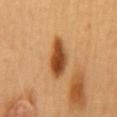No biopsy was performed on this lesion — it was imaged during a full skin examination and was not determined to be concerning.
The total-body-photography lesion software estimated a mean CIELAB color near L≈50 a*≈27 b*≈42 and a lesion-to-skin contrast of about 12 (normalized; higher = more distinct). The software also gave a border-irregularity index near 2/10 and a color-variation rating of about 5.5/10. The software also gave a nevus-likeness score of about 95/100 and a detector confidence of about 100 out of 100 that the crop contains a lesion.
The recorded lesion diameter is about 5 mm.
A female patient approximately 60 years of age.
Imaged with cross-polarized lighting.
Located on the mid back.
A roughly 15 mm field-of-view crop from a total-body skin photograph.